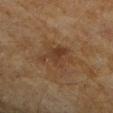The lesion was tiled from a total-body skin photograph and was not biopsied.
A close-up tile cropped from a whole-body skin photograph, about 15 mm across.
A female patient aged approximately 60.
The tile uses cross-polarized illumination.
On the right forearm.